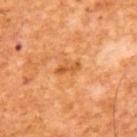This lesion was catalogued during total-body skin photography and was not selected for biopsy.
The recorded lesion diameter is about 3 mm.
A 15 mm close-up extracted from a 3D total-body photography capture.
The tile uses cross-polarized illumination.
A male patient in their mid-60s.
The total-body-photography lesion software estimated an outline eccentricity of about 0.9 (0 = round, 1 = elongated).